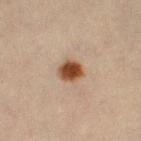The lesion was tiled from a total-body skin photograph and was not biopsied.
The subject is a female approximately 45 years of age.
The lesion's longest dimension is about 3 mm.
On the left thigh.
An algorithmic analysis of the crop reported a lesion area of about 6 mm², an outline eccentricity of about 0.45 (0 = round, 1 = elongated), and a symmetry-axis asymmetry near 0.2. The software also gave an average lesion color of about L≈42 a*≈18 b*≈30 (CIELAB), about 15 CIELAB-L* units darker than the surrounding skin, and a normalized lesion–skin contrast near 12.5. The analysis additionally found a border-irregularity rating of about 1.5/10, a color-variation rating of about 4.5/10, and a peripheral color-asymmetry measure near 1.5.
A 15 mm close-up extracted from a 3D total-body photography capture.